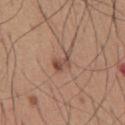Approximately 3.5 mm at its widest.
The lesion is located on the chest.
A male patient approximately 65 years of age.
Automated image analysis of the tile measured about 9 CIELAB-L* units darker than the surrounding skin and a lesion-to-skin contrast of about 6.5 (normalized; higher = more distinct). It also reported a border-irregularity rating of about 4/10 and a within-lesion color-variation index near 4/10.
This is a white-light tile.
A lesion tile, about 15 mm wide, cut from a 3D total-body photograph.